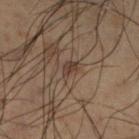{
  "biopsy_status": "not biopsied; imaged during a skin examination",
  "site": "left lower leg",
  "lighting": "cross-polarized",
  "lesion_size": {
    "long_diameter_mm_approx": 2.5
  },
  "image": {
    "source": "total-body photography crop",
    "field_of_view_mm": 15
  },
  "automated_metrics": {
    "nevus_likeness_0_100": 5,
    "lesion_detection_confidence_0_100": 75
  },
  "patient": {
    "sex": "male",
    "age_approx": 50
  }
}